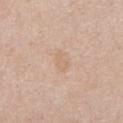| field | value |
|---|---|
| body site | the chest |
| tile lighting | white-light illumination |
| imaging modality | ~15 mm crop, total-body skin-cancer survey |
| patient | male, about 55 years old |
| size | about 2.5 mm |